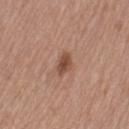  biopsy_status: not biopsied; imaged during a skin examination
  site: left thigh
  lighting: white-light
  automated_metrics:
    area_mm2_approx: 4.0
    eccentricity: 0.7
    shape_asymmetry: 0.25
    cielab_L: 48
    cielab_a: 22
    cielab_b: 29
    vs_skin_darker_L: 11.0
    vs_skin_contrast_norm: 8.5
    border_irregularity_0_10: 2.0
    color_variation_0_10: 2.0
    peripheral_color_asymmetry: 0.5
    nevus_likeness_0_100: 90
    lesion_detection_confidence_0_100: 100
  patient:
    sex: female
    age_approx: 55
  image:
    source: total-body photography crop
    field_of_view_mm: 15